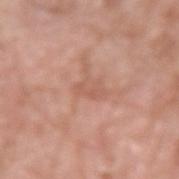The lesion was photographed on a routine skin check and not biopsied; there is no pathology result. A 15 mm close-up tile from a total-body photography series done for melanoma screening. The patient is a male roughly 60 years of age. The recorded lesion diameter is about 2.5 mm. From the left upper arm.